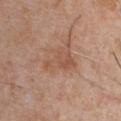No biopsy was performed on this lesion — it was imaged during a full skin examination and was not determined to be concerning. The recorded lesion diameter is about 4.5 mm. Imaged with white-light lighting. Located on the chest. Cropped from a total-body skin-imaging series; the visible field is about 15 mm. A male subject aged 28–32.